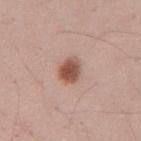No biopsy was performed on this lesion — it was imaged during a full skin examination and was not determined to be concerning. Longest diameter approximately 3 mm. A male patient, aged approximately 25. The lesion is on the abdomen. Cropped from a total-body skin-imaging series; the visible field is about 15 mm. The tile uses white-light illumination.